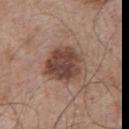Q: Was this lesion biopsied?
A: imaged on a skin check; not biopsied
Q: Patient demographics?
A: male, approximately 65 years of age
Q: What kind of image is this?
A: ~15 mm tile from a whole-body skin photo
Q: What lighting was used for the tile?
A: white-light
Q: What is the anatomic site?
A: the chest
Q: How large is the lesion?
A: about 4.5 mm